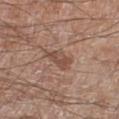Assessment: Recorded during total-body skin imaging; not selected for excision or biopsy. Context: Located on the right lower leg. Automated tile analysis of the lesion measured an area of roughly 5.5 mm², an outline eccentricity of about 0.9 (0 = round, 1 = elongated), and a shape-asymmetry score of about 0.35 (0 = symmetric). The software also gave a color-variation rating of about 2.5/10 and radial color variation of about 0.5. Imaged with white-light lighting. Cropped from a total-body skin-imaging series; the visible field is about 15 mm. The subject is a male aged 58 to 62.